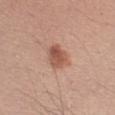* follow-up — total-body-photography surveillance lesion; no biopsy
* anatomic site — the arm
* lighting — white-light illumination
* subject — male, roughly 30 years of age
* image source — 15 mm crop, total-body photography
* diameter — ≈3.5 mm